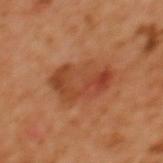Assessment:
The lesion was tiled from a total-body skin photograph and was not biopsied.
Clinical summary:
The subject is a female about 40 years old. A 15 mm close-up tile from a total-body photography series done for melanoma screening. Imaged with cross-polarized lighting. The lesion is located on the upper back.